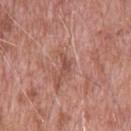biopsy status: imaged on a skin check; not biopsied | imaging modality: 15 mm crop, total-body photography | body site: the upper back | patient: male, in their 50s | lesion diameter: ~3 mm (longest diameter).